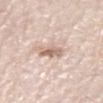Q: Was a biopsy performed?
A: no biopsy performed (imaged during a skin exam)
Q: What are the patient's age and sex?
A: male, about 80 years old
Q: What is the anatomic site?
A: the right thigh
Q: What is the imaging modality?
A: ~15 mm crop, total-body skin-cancer survey